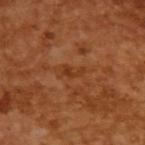{
  "biopsy_status": "not biopsied; imaged during a skin examination",
  "lesion_size": {
    "long_diameter_mm_approx": 2.5
  },
  "patient": {
    "sex": "male",
    "age_approx": 65
  },
  "image": {
    "source": "total-body photography crop",
    "field_of_view_mm": 15
  },
  "lighting": "cross-polarized"
}